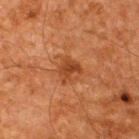Background:
A male subject, aged 58 to 62. This is a cross-polarized tile. The lesion's longest dimension is about 2.5 mm. A close-up tile cropped from a whole-body skin photograph, about 15 mm across. From the upper back.